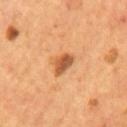Case summary:
- biopsy status · total-body-photography surveillance lesion; no biopsy
- lesion size · about 3.5 mm
- image · ~15 mm crop, total-body skin-cancer survey
- illumination · cross-polarized
- location · the mid back
- patient · male, in their mid- to late 50s